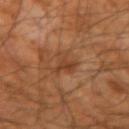Imaged during a routine full-body skin examination; the lesion was not biopsied and no histopathology is available.
The lesion is on the left forearm.
Automated tile analysis of the lesion measured a footprint of about 5.5 mm², an eccentricity of roughly 0.8, and two-axis asymmetry of about 0.55. The software also gave about 7 CIELAB-L* units darker than the surrounding skin and a normalized border contrast of about 6. It also reported a nevus-likeness score of about 0/100 and lesion-presence confidence of about 85/100.
Cropped from a total-body skin-imaging series; the visible field is about 15 mm.
The patient is a male roughly 60 years of age.
Captured under cross-polarized illumination.